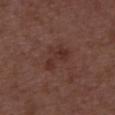biopsy status: imaged on a skin check; not biopsied | TBP lesion metrics: a border-irregularity index near 6.5/10, a color-variation rating of about 1.5/10, and a peripheral color-asymmetry measure near 0.5 | patient: male, in their 50s | image: ~15 mm tile from a whole-body skin photo | diameter: ~4 mm (longest diameter) | tile lighting: white-light.